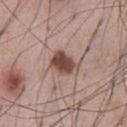The lesion was photographed on a routine skin check and not biopsied; there is no pathology result. About 3.5 mm across. A male patient, in their mid-50s. The lesion is located on the abdomen. A 15 mm crop from a total-body photograph taken for skin-cancer surveillance. The tile uses white-light illumination.